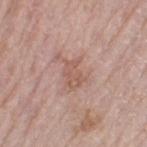<case>
  <automated_metrics>
    <nevus_likeness_0_100>0</nevus_likeness_0_100>
  </automated_metrics>
  <site>left thigh</site>
  <patient>
    <sex>female</sex>
    <age_approx>65</age_approx>
  </patient>
  <lesion_size>
    <long_diameter_mm_approx>3.5</long_diameter_mm_approx>
  </lesion_size>
  <image>
    <source>total-body photography crop</source>
    <field_of_view_mm>15</field_of_view_mm>
  </image>
</case>